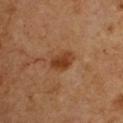biopsy status: imaged on a skin check; not biopsied
anatomic site: the chest
tile lighting: cross-polarized
lesion diameter: ≈3 mm
image source: ~15 mm crop, total-body skin-cancer survey
patient: male, aged 48 to 52
image-analysis metrics: a lesion area of about 5.5 mm², an outline eccentricity of about 0.75 (0 = round, 1 = elongated), and a symmetry-axis asymmetry near 0.2; a lesion–skin lightness drop of about 10 and a normalized border contrast of about 9; internal color variation of about 3.5 on a 0–10 scale